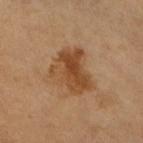biopsy_status: not biopsied; imaged during a skin examination
image:
  source: total-body photography crop
  field_of_view_mm: 15
patient:
  sex: female
  age_approx: 55
site: right lower leg
lesion_size:
  long_diameter_mm_approx: 5.5